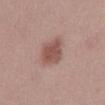imaging modality = total-body-photography crop, ~15 mm field of view
site = the lower back
subject = female, aged 23–27
automated lesion analysis = a border-irregularity rating of about 2.5/10, a color-variation rating of about 2.5/10, and a peripheral color-asymmetry measure near 1
illumination = white-light
lesion diameter = ~4.5 mm (longest diameter)